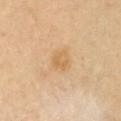– notes — total-body-photography surveillance lesion; no biopsy
– site — the chest
– imaging modality — 15 mm crop, total-body photography
– automated metrics — a lesion area of about 4.5 mm² and a shape eccentricity near 0.5; an average lesion color of about L≈66 a*≈17 b*≈42 (CIELAB) and a lesion–skin lightness drop of about 7; a nevus-likeness score of about 0/100 and lesion-presence confidence of about 100/100
– illumination — cross-polarized
– subject — female, aged 58 to 62